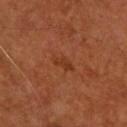Captured during whole-body skin photography for melanoma surveillance; the lesion was not biopsied.
A 15 mm close-up extracted from a 3D total-body photography capture.
Approximately 2.5 mm at its widest.
The lesion is on the upper back.
An algorithmic analysis of the crop reported a lesion color around L≈32 a*≈24 b*≈32 in CIELAB and a lesion–skin lightness drop of about 6.
This is a cross-polarized tile.
A male patient roughly 55 years of age.